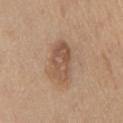Impression:
The lesion was photographed on a routine skin check and not biopsied; there is no pathology result.
Background:
A roughly 15 mm field-of-view crop from a total-body skin photograph. On the chest. Automated image analysis of the tile measured two-axis asymmetry of about 0.2. It also reported a mean CIELAB color near L≈53 a*≈18 b*≈30, a lesion–skin lightness drop of about 10, and a normalized border contrast of about 7. The patient is a male roughly 60 years of age. The tile uses white-light illumination.